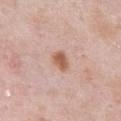Part of a total-body skin-imaging series; this lesion was reviewed on a skin check and was not flagged for biopsy.
Captured under white-light illumination.
The lesion is on the abdomen.
This image is a 15 mm lesion crop taken from a total-body photograph.
A male patient, in their mid- to late 50s.